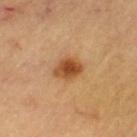Findings:
• workup — no biopsy performed (imaged during a skin exam)